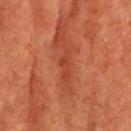Clinical impression: No biopsy was performed on this lesion — it was imaged during a full skin examination and was not determined to be concerning. Image and clinical context: The total-body-photography lesion software estimated a lesion–skin lightness drop of about 7. And it measured a border-irregularity rating of about 6/10, a color-variation rating of about 0/10, and peripheral color asymmetry of about 0. It also reported an automated nevus-likeness rating near 0 out of 100 and a lesion-detection confidence of about 80/100. A male subject, aged 68–72. Captured under cross-polarized illumination. The lesion is on the head or neck. Approximately 3.5 mm at its widest. A 15 mm crop from a total-body photograph taken for skin-cancer surveillance.